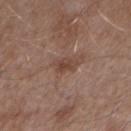automated metrics: an area of roughly 3.5 mm², a shape eccentricity near 0.8, and two-axis asymmetry of about 0.35; a mean CIELAB color near L≈43 a*≈19 b*≈25, a lesion–skin lightness drop of about 8, and a lesion-to-skin contrast of about 6.5 (normalized; higher = more distinct); border irregularity of about 4 on a 0–10 scale, a color-variation rating of about 1.5/10, and peripheral color asymmetry of about 0.5 | patient: male, aged approximately 55 | tile lighting: white-light illumination | size: about 2.5 mm | imaging modality: ~15 mm tile from a whole-body skin photo | body site: the arm.